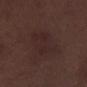Captured during whole-body skin photography for melanoma surveillance; the lesion was not biopsied.